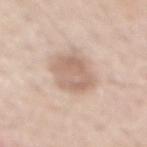Assessment: No biopsy was performed on this lesion — it was imaged during a full skin examination and was not determined to be concerning. Acquisition and patient details: The lesion-visualizer software estimated about 11 CIELAB-L* units darker than the surrounding skin and a lesion-to-skin contrast of about 6.5 (normalized; higher = more distinct). This is a white-light tile. From the mid back. A male subject aged around 55. A region of skin cropped from a whole-body photographic capture, roughly 15 mm wide.